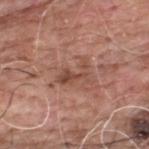Notes:
• biopsy status · imaged on a skin check; not biopsied
• subject · male, aged around 60
• lighting · white-light
• size · ≈4 mm
• image-analysis metrics · a shape eccentricity near 0.85 and a symmetry-axis asymmetry near 0.5
• anatomic site · the upper back
• imaging modality · total-body-photography crop, ~15 mm field of view